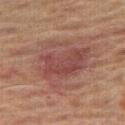Q: Who is the patient?
A: male, roughly 65 years of age
Q: How was this image acquired?
A: total-body-photography crop, ~15 mm field of view
Q: Illumination type?
A: cross-polarized illumination
Q: What is the anatomic site?
A: the right thigh
Q: How large is the lesion?
A: ≈7 mm